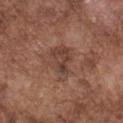Assessment: The lesion was tiled from a total-body skin photograph and was not biopsied. Clinical summary: The patient is a male approximately 75 years of age. Captured under white-light illumination. A 15 mm close-up extracted from a 3D total-body photography capture. The lesion is on the chest. Measured at roughly 4.5 mm in maximum diameter.